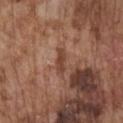Case summary:
– workup · imaged on a skin check; not biopsied
– tile lighting · white-light
– body site · the chest
– acquisition · ~15 mm tile from a whole-body skin photo
– lesion diameter · ≈3 mm
– subject · male, aged approximately 75